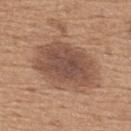Impression: The lesion was photographed on a routine skin check and not biopsied; there is no pathology result. Background: Automated tile analysis of the lesion measured a footprint of about 31 mm² and an eccentricity of roughly 0.75. The software also gave border irregularity of about 2.5 on a 0–10 scale and radial color variation of about 1. The software also gave a classifier nevus-likeness of about 50/100 and a lesion-detection confidence of about 100/100. A region of skin cropped from a whole-body photographic capture, roughly 15 mm wide. Located on the upper back. A male patient, aged 63–67. The tile uses white-light illumination.